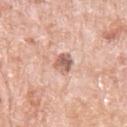The lesion was photographed on a routine skin check and not biopsied; there is no pathology result.
On the left upper arm.
Automated tile analysis of the lesion measured roughly 14 lightness units darker than nearby skin and a normalized lesion–skin contrast near 8.5. The analysis additionally found a border-irregularity rating of about 2/10 and a color-variation rating of about 5.5/10. It also reported a classifier nevus-likeness of about 0/100 and a detector confidence of about 100 out of 100 that the crop contains a lesion.
A 15 mm crop from a total-body photograph taken for skin-cancer surveillance.
A male patient aged around 80.
This is a white-light tile.
The recorded lesion diameter is about 2.5 mm.